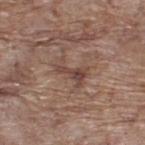image source: 15 mm crop, total-body photography
illumination: white-light
anatomic site: the upper back
size: ~4 mm (longest diameter)
subject: male, aged approximately 70